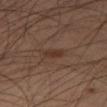Image and clinical context:
Longest diameter approximately 2.5 mm. Captured under cross-polarized illumination. Cropped from a whole-body photographic skin survey; the tile spans about 15 mm. A male patient, in their mid-50s. Located on the right thigh.